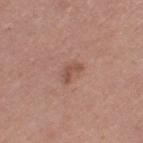biopsy_status: not biopsied; imaged during a skin examination
image:
  source: total-body photography crop
  field_of_view_mm: 15
patient:
  sex: female
  age_approx: 30
lesion_size:
  long_diameter_mm_approx: 2.5
site: leg
lighting: white-light
automated_metrics:
  vs_skin_contrast_norm: 6.5
  nevus_likeness_0_100: 20
  lesion_detection_confidence_0_100: 100